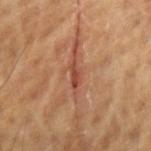The lesion was photographed on a routine skin check and not biopsied; there is no pathology result. Located on the left forearm. A 15 mm close-up extracted from a 3D total-body photography capture. The subject is a male roughly 60 years of age.